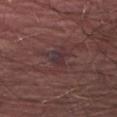Impression:
The lesion was photographed on a routine skin check and not biopsied; there is no pathology result.
Image and clinical context:
Longest diameter approximately 3.5 mm. A male subject, aged 38 to 42. A 15 mm close-up extracted from a 3D total-body photography capture. Automated tile analysis of the lesion measured an automated nevus-likeness rating near 0 out of 100 and a detector confidence of about 85 out of 100 that the crop contains a lesion. The lesion is on the abdomen.